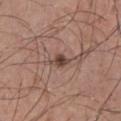Recorded during total-body skin imaging; not selected for excision or biopsy. A male patient, aged 28–32. The lesion is on the left lower leg. The lesion-visualizer software estimated a footprint of about 3.5 mm², a shape eccentricity near 0.85, and a shape-asymmetry score of about 0.4 (0 = symmetric). And it measured a mean CIELAB color near L≈44 a*≈18 b*≈23 and about 12 CIELAB-L* units darker than the surrounding skin. A 15 mm close-up extracted from a 3D total-body photography capture. Approximately 3 mm at its widest.